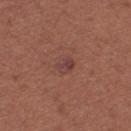Notes:
- workup · no biopsy performed (imaged during a skin exam)
- body site · the left thigh
- patient · female, roughly 35 years of age
- image · ~15 mm tile from a whole-body skin photo